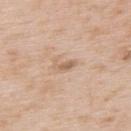This lesion was catalogued during total-body skin photography and was not selected for biopsy.
A 15 mm close-up extracted from a 3D total-body photography capture.
The lesion is located on the back.
A male subject, aged 63 to 67.